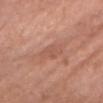Findings:
- follow-up — total-body-photography surveillance lesion; no biopsy
- illumination — white-light
- subject — female, aged 73–77
- automated lesion analysis — a footprint of about 5.5 mm² and a shape eccentricity near 0.85; about 6 CIELAB-L* units darker than the surrounding skin; a border-irregularity index near 3/10 and radial color variation of about 0.5; an automated nevus-likeness rating near 0 out of 100 and a detector confidence of about 95 out of 100 that the crop contains a lesion
- image source — total-body-photography crop, ~15 mm field of view
- lesion size — ~3.5 mm (longest diameter)
- body site — the head or neck